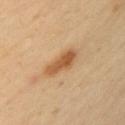No biopsy was performed on this lesion — it was imaged during a full skin examination and was not determined to be concerning. This image is a 15 mm lesion crop taken from a total-body photograph. From the left upper arm. The recorded lesion diameter is about 4 mm. The subject is a female roughly 45 years of age. Captured under cross-polarized illumination.